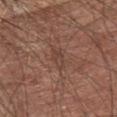Impression: This lesion was catalogued during total-body skin photography and was not selected for biopsy. Clinical summary: Located on the front of the torso. Cropped from a whole-body photographic skin survey; the tile spans about 15 mm. The subject is a female aged around 65.